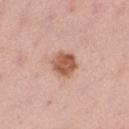– notes: catalogued during a skin exam; not biopsied